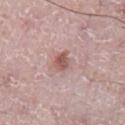biopsy status: total-body-photography surveillance lesion; no biopsy | lighting: white-light illumination | automated metrics: border irregularity of about 2.5 on a 0–10 scale and a color-variation rating of about 3.5/10 | diameter: ≈2.5 mm | image source: 15 mm crop, total-body photography | subject: male, roughly 75 years of age | body site: the abdomen.